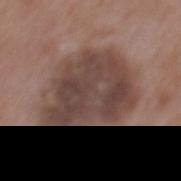Part of a total-body skin-imaging series; this lesion was reviewed on a skin check and was not flagged for biopsy.
A 15 mm close-up tile from a total-body photography series done for melanoma screening.
From the mid back.
The lesion-visualizer software estimated an eccentricity of roughly 0.6 and a shape-asymmetry score of about 0.2 (0 = symmetric).
The tile uses white-light illumination.
A male patient, aged 63 to 67.
Measured at roughly 9 mm in maximum diameter.